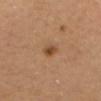Assessment: Part of a total-body skin-imaging series; this lesion was reviewed on a skin check and was not flagged for biopsy. Acquisition and patient details: Approximately 2 mm at its widest. A female patient aged around 35. On the head or neck. An algorithmic analysis of the crop reported a lesion area of about 3 mm² and two-axis asymmetry of about 0.2. The analysis additionally found a lesion color around L≈47 a*≈21 b*≈34 in CIELAB and a normalized border contrast of about 8. It also reported a border-irregularity rating of about 2/10, a within-lesion color-variation index near 2.5/10, and peripheral color asymmetry of about 1. It also reported a classifier nevus-likeness of about 90/100 and lesion-presence confidence of about 100/100. This is a cross-polarized tile. This image is a 15 mm lesion crop taken from a total-body photograph.